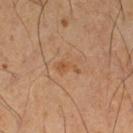Impression:
No biopsy was performed on this lesion — it was imaged during a full skin examination and was not determined to be concerning.
Clinical summary:
Longest diameter approximately 3 mm. The lesion is located on the left thigh. Cropped from a whole-body photographic skin survey; the tile spans about 15 mm. An algorithmic analysis of the crop reported a nevus-likeness score of about 0/100 and a lesion-detection confidence of about 100/100. Captured under cross-polarized illumination. A male subject aged 58–62.